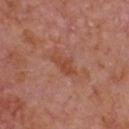Q: Automated lesion metrics?
A: a shape eccentricity near 0.85 and two-axis asymmetry of about 0.35; a mean CIELAB color near L≈47 a*≈26 b*≈32 and a lesion-to-skin contrast of about 6 (normalized; higher = more distinct); radial color variation of about 0.5; a detector confidence of about 100 out of 100 that the crop contains a lesion
Q: Lesion location?
A: the chest
Q: Lesion size?
A: ≈3.5 mm
Q: Who is the patient?
A: male, roughly 65 years of age
Q: What kind of image is this?
A: total-body-photography crop, ~15 mm field of view
Q: Illumination type?
A: white-light illumination